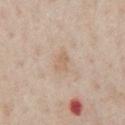Impression: This lesion was catalogued during total-body skin photography and was not selected for biopsy. Context: Approximately 2.5 mm at its widest. This is a white-light tile. Cropped from a total-body skin-imaging series; the visible field is about 15 mm. The subject is a male aged approximately 60. The lesion is on the chest.